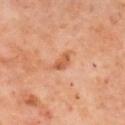Q: Was a biopsy performed?
A: catalogued during a skin exam; not biopsied
Q: Patient demographics?
A: male, aged 58–62
Q: What is the imaging modality?
A: 15 mm crop, total-body photography
Q: Lesion location?
A: the mid back
Q: What lighting was used for the tile?
A: cross-polarized